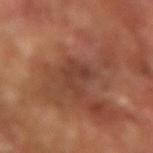  biopsy_status: not biopsied; imaged during a skin examination
  patient:
    sex: male
    age_approx: 65
  site: arm
  image:
    source: total-body photography crop
    field_of_view_mm: 15